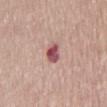Q: Was a biopsy performed?
A: total-body-photography surveillance lesion; no biopsy
Q: What lighting was used for the tile?
A: white-light illumination
Q: What is the imaging modality?
A: ~15 mm tile from a whole-body skin photo
Q: How large is the lesion?
A: about 3 mm
Q: Patient demographics?
A: male, aged around 75
Q: Lesion location?
A: the abdomen